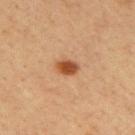Assessment: Recorded during total-body skin imaging; not selected for excision or biopsy. Acquisition and patient details: From the upper back. An algorithmic analysis of the crop reported a mean CIELAB color near L≈45 a*≈23 b*≈35, roughly 13 lightness units darker than nearby skin, and a normalized border contrast of about 10. It also reported an automated nevus-likeness rating near 100 out of 100 and lesion-presence confidence of about 100/100. The recorded lesion diameter is about 2.5 mm. Cropped from a whole-body photographic skin survey; the tile spans about 15 mm. Captured under cross-polarized illumination. The subject is a male in their 40s.